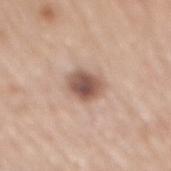<lesion>
<biopsy_status>not biopsied; imaged during a skin examination</biopsy_status>
<lighting>white-light</lighting>
<patient>
  <sex>male</sex>
  <age_approx>70</age_approx>
</patient>
<image>
  <source>total-body photography crop</source>
  <field_of_view_mm>15</field_of_view_mm>
</image>
<lesion_size>
  <long_diameter_mm_approx>3.5</long_diameter_mm_approx>
</lesion_size>
<site>mid back</site>
</lesion>